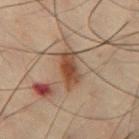| feature | finding |
|---|---|
| patient | male, aged approximately 50 |
| image | ~15 mm crop, total-body skin-cancer survey |
| location | the chest |
| illumination | cross-polarized |
| lesion diameter | about 4.5 mm |